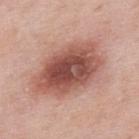<record>
<biopsy_status>not biopsied; imaged during a skin examination</biopsy_status>
<patient>
  <sex>male</sex>
  <age_approx>55</age_approx>
</patient>
<automated_metrics>
  <cielab_L>52</cielab_L>
  <cielab_a>25</cielab_a>
  <cielab_b>26</cielab_b>
  <vs_skin_darker_L>16.0</vs_skin_darker_L>
  <border_irregularity_0_10>2.0</border_irregularity_0_10>
  <peripheral_color_asymmetry>2.0</peripheral_color_asymmetry>
</automated_metrics>
<image>
  <source>total-body photography crop</source>
  <field_of_view_mm>15</field_of_view_mm>
</image>
<site>upper back</site>
<lighting>white-light</lighting>
<lesion_size>
  <long_diameter_mm_approx>8.5</long_diameter_mm_approx>
</lesion_size>
</record>